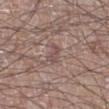| field | value |
|---|---|
| diameter | ≈2.5 mm |
| location | the left lower leg |
| illumination | white-light |
| subject | male, in their 60s |
| image source | total-body-photography crop, ~15 mm field of view |